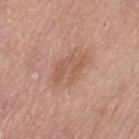Captured during whole-body skin photography for melanoma surveillance; the lesion was not biopsied.
A roughly 15 mm field-of-view crop from a total-body skin photograph.
From the leg.
The tile uses white-light illumination.
Automated tile analysis of the lesion measured border irregularity of about 4.5 on a 0–10 scale, a color-variation rating of about 1.5/10, and peripheral color asymmetry of about 0.5. The software also gave a lesion-detection confidence of about 100/100.
The subject is a female aged approximately 65.
Approximately 5 mm at its widest.